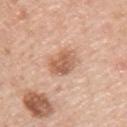Acquisition and patient details: A male subject in their 40s. The total-body-photography lesion software estimated a classifier nevus-likeness of about 95/100. Located on the arm. A roughly 15 mm field-of-view crop from a total-body skin photograph. The lesion's longest dimension is about 4.5 mm.